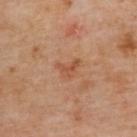automated metrics=a footprint of about 3.5 mm² and an eccentricity of roughly 0.7; an average lesion color of about L≈51 a*≈24 b*≈33 (CIELAB), a lesion–skin lightness drop of about 8, and a normalized border contrast of about 6; an automated nevus-likeness rating near 0 out of 100 and a detector confidence of about 100 out of 100 that the crop contains a lesion | patient=female, about 60 years old | acquisition=~15 mm tile from a whole-body skin photo | anatomic site=the back | lesion size=about 3 mm.